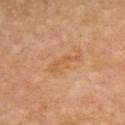Q: Is there a histopathology result?
A: imaged on a skin check; not biopsied
Q: How large is the lesion?
A: about 4 mm
Q: What is the anatomic site?
A: the upper back
Q: How was the tile lit?
A: cross-polarized
Q: What are the patient's age and sex?
A: female, about 65 years old
Q: What kind of image is this?
A: 15 mm crop, total-body photography